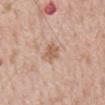Recorded during total-body skin imaging; not selected for excision or biopsy. From the mid back. Captured under white-light illumination. The lesion's longest dimension is about 3 mm. A male subject, about 50 years old. This image is a 15 mm lesion crop taken from a total-body photograph.